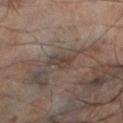lighting: cross-polarized
patient:
  sex: male
  age_approx: 55
image:
  source: total-body photography crop
  field_of_view_mm: 15
site: left lower leg